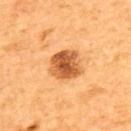Case summary:
• biopsy status: imaged on a skin check; not biopsied
• site: the upper back
• imaging modality: ~15 mm tile from a whole-body skin photo
• TBP lesion metrics: an area of roughly 11 mm², an eccentricity of roughly 0.35, and a shape-asymmetry score of about 0.15 (0 = symmetric); an average lesion color of about L≈57 a*≈28 b*≈44 (CIELAB), about 17 CIELAB-L* units darker than the surrounding skin, and a lesion-to-skin contrast of about 10 (normalized; higher = more distinct)
• diameter: ~4 mm (longest diameter)
• patient: male, aged approximately 65